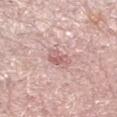Acquisition and patient details: The lesion is on the left lower leg. Cropped from a total-body skin-imaging series; the visible field is about 15 mm. About 3 mm across. A female subject, in their mid-60s.